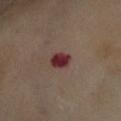Clinical impression: No biopsy was performed on this lesion — it was imaged during a full skin examination and was not determined to be concerning. Acquisition and patient details: Captured under cross-polarized illumination. A close-up tile cropped from a whole-body skin photograph, about 15 mm across. A female patient approximately 55 years of age. The recorded lesion diameter is about 2.5 mm. On the abdomen.